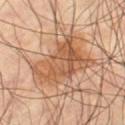<tbp_lesion>
<image>
  <source>total-body photography crop</source>
  <field_of_view_mm>15</field_of_view_mm>
</image>
<lighting>cross-polarized</lighting>
<site>leg</site>
<patient>
  <sex>male</sex>
  <age_approx>60</age_approx>
</patient>
<lesion_size>
  <long_diameter_mm_approx>6.5</long_diameter_mm_approx>
</lesion_size>
<automated_metrics>
  <eccentricity>0.65</eccentricity>
  <shape_asymmetry>0.4</shape_asymmetry>
  <cielab_L>55</cielab_L>
  <cielab_a>22</cielab_a>
  <cielab_b>35</cielab_b>
  <vs_skin_darker_L>10.0</vs_skin_darker_L>
  <border_irregularity_0_10>5.0</border_irregularity_0_10>
  <color_variation_0_10>6.5</color_variation_0_10>
  <peripheral_color_asymmetry>2.5</peripheral_color_asymmetry>
</automated_metrics>
</tbp_lesion>